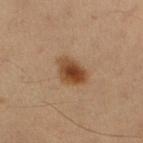Case summary:
– notes: total-body-photography surveillance lesion; no biopsy
– acquisition: ~15 mm crop, total-body skin-cancer survey
– size: ~3.5 mm (longest diameter)
– automated lesion analysis: a footprint of about 8 mm² and two-axis asymmetry of about 0.2; border irregularity of about 2 on a 0–10 scale, a color-variation rating of about 4.5/10, and a peripheral color-asymmetry measure near 1; a classifier nevus-likeness of about 100/100 and a lesion-detection confidence of about 100/100
– site: the arm
– subject: male, aged around 55